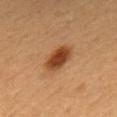| feature | finding |
|---|---|
| notes | catalogued during a skin exam; not biopsied |
| location | the mid back |
| diameter | ≈4 mm |
| illumination | cross-polarized |
| patient | male, aged approximately 60 |
| image-analysis metrics | a lesion area of about 8.5 mm², an eccentricity of roughly 0.8, and a symmetry-axis asymmetry near 0.1; border irregularity of about 1.5 on a 0–10 scale and a within-lesion color-variation index near 4/10 |
| image source | ~15 mm crop, total-body skin-cancer survey |